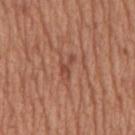This lesion was catalogued during total-body skin photography and was not selected for biopsy.
Cropped from a total-body skin-imaging series; the visible field is about 15 mm.
The lesion-visualizer software estimated a lesion area of about 3 mm² and an outline eccentricity of about 0.85 (0 = round, 1 = elongated). The analysis additionally found an average lesion color of about L≈47 a*≈25 b*≈30 (CIELAB), a lesion–skin lightness drop of about 8, and a lesion-to-skin contrast of about 5.5 (normalized; higher = more distinct). And it measured border irregularity of about 6.5 on a 0–10 scale and peripheral color asymmetry of about 0. The software also gave a classifier nevus-likeness of about 0/100 and a lesion-detection confidence of about 90/100.
Measured at roughly 3 mm in maximum diameter.
Located on the mid back.
A male patient, aged around 65.
This is a white-light tile.